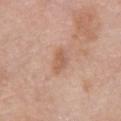Clinical impression: The lesion was tiled from a total-body skin photograph and was not biopsied. Image and clinical context: A close-up tile cropped from a whole-body skin photograph, about 15 mm across. Longest diameter approximately 3 mm. This is a white-light tile. The total-body-photography lesion software estimated a lesion area of about 4.5 mm², an outline eccentricity of about 0.8 (0 = round, 1 = elongated), and a symmetry-axis asymmetry near 0.3. And it measured a normalized border contrast of about 6. The analysis additionally found border irregularity of about 2.5 on a 0–10 scale, internal color variation of about 1.5 on a 0–10 scale, and a peripheral color-asymmetry measure near 0.5. The lesion is located on the chest. A female patient, about 65 years old.